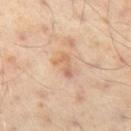Q: Was a biopsy performed?
A: catalogued during a skin exam; not biopsied
Q: How large is the lesion?
A: about 3 mm
Q: What is the anatomic site?
A: the left thigh
Q: Automated lesion metrics?
A: an automated nevus-likeness rating near 0 out of 100 and a detector confidence of about 100 out of 100 that the crop contains a lesion
Q: How was the tile lit?
A: cross-polarized illumination
Q: What kind of image is this?
A: ~15 mm crop, total-body skin-cancer survey
Q: What are the patient's age and sex?
A: male, aged around 50